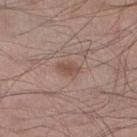{
  "biopsy_status": "not biopsied; imaged during a skin examination",
  "site": "right lower leg",
  "lighting": "white-light",
  "automated_metrics": {
    "area_mm2_approx": 4.0,
    "eccentricity": 0.8,
    "shape_asymmetry": 0.25
  },
  "lesion_size": {
    "long_diameter_mm_approx": 3.0
  },
  "patient": {
    "sex": "male",
    "age_approx": 55
  },
  "image": {
    "source": "total-body photography crop",
    "field_of_view_mm": 15
  }
}